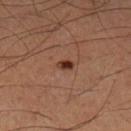Q: Was a biopsy performed?
A: total-body-photography surveillance lesion; no biopsy
Q: What kind of image is this?
A: total-body-photography crop, ~15 mm field of view
Q: Who is the patient?
A: male, aged 48–52
Q: Lesion location?
A: the left lower leg